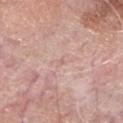Recorded during total-body skin imaging; not selected for excision or biopsy. The tile uses white-light illumination. Located on the head or neck. Longest diameter approximately 1 mm. A male patient aged around 70. Automated tile analysis of the lesion measured a mean CIELAB color near L≈64 a*≈21 b*≈24. The analysis additionally found a border-irregularity rating of about 3.5/10. A close-up tile cropped from a whole-body skin photograph, about 15 mm across.